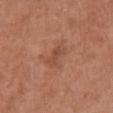The lesion-visualizer software estimated a shape eccentricity near 0.85 and a shape-asymmetry score of about 0.3 (0 = symmetric). The software also gave a mean CIELAB color near L≈48 a*≈25 b*≈31, about 7 CIELAB-L* units darker than the surrounding skin, and a normalized border contrast of about 5.5. It also reported an automated nevus-likeness rating near 0 out of 100 and lesion-presence confidence of about 100/100. Cropped from a total-body skin-imaging series; the visible field is about 15 mm. The lesion is on the chest. The patient is a female aged 63–67.